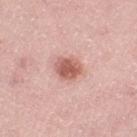Part of a total-body skin-imaging series; this lesion was reviewed on a skin check and was not flagged for biopsy. A roughly 15 mm field-of-view crop from a total-body skin photograph. Captured under white-light illumination. The patient is a female approximately 40 years of age. On the left thigh.